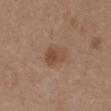This lesion was catalogued during total-body skin photography and was not selected for biopsy. Measured at roughly 3 mm in maximum diameter. The lesion is on the front of the torso. Captured under white-light illumination. The subject is a female in their 30s. Cropped from a whole-body photographic skin survey; the tile spans about 15 mm.